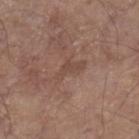• patient: male, about 80 years old
• size: about 4.5 mm
• site: the right lower leg
• automated metrics: an average lesion color of about L≈46 a*≈18 b*≈24 (CIELAB), about 6 CIELAB-L* units darker than the surrounding skin, and a lesion-to-skin contrast of about 5 (normalized; higher = more distinct); a detector confidence of about 55 out of 100 that the crop contains a lesion
• imaging modality: ~15 mm crop, total-body skin-cancer survey